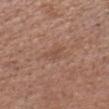patient = male, aged approximately 50; illumination = white-light; location = the head or neck; acquisition = 15 mm crop, total-body photography; diameter = ≈2.5 mm.